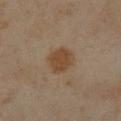workup: catalogued during a skin exam; not biopsied
lesion diameter: about 3.5 mm
automated metrics: an area of roughly 8.5 mm², a shape eccentricity near 0.5, and a symmetry-axis asymmetry near 0.15; an average lesion color of about L≈42 a*≈16 b*≈30 (CIELAB) and about 8 CIELAB-L* units darker than the surrounding skin; border irregularity of about 1.5 on a 0–10 scale and a peripheral color-asymmetry measure near 0.5; a classifier nevus-likeness of about 95/100
acquisition: ~15 mm crop, total-body skin-cancer survey
location: the left forearm
patient: female, aged approximately 35